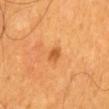<tbp_lesion>
  <biopsy_status>not biopsied; imaged during a skin examination</biopsy_status>
  <patient>
    <sex>male</sex>
    <age_approx>60</age_approx>
  </patient>
  <image>
    <source>total-body photography crop</source>
    <field_of_view_mm>15</field_of_view_mm>
  </image>
  <site>mid back</site>
</tbp_lesion>